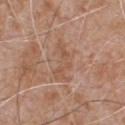notes=total-body-photography surveillance lesion; no biopsy
body site=the chest
image source=total-body-photography crop, ~15 mm field of view
patient=male, roughly 65 years of age